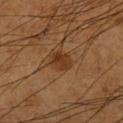workup — total-body-photography surveillance lesion; no biopsy
anatomic site — the left forearm
acquisition — total-body-photography crop, ~15 mm field of view
patient — male, aged approximately 60